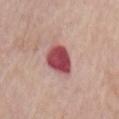follow-up: catalogued during a skin exam; not biopsied
imaging modality: ~15 mm crop, total-body skin-cancer survey
site: the arm
lesion size: about 4 mm
TBP lesion metrics: an area of roughly 10 mm² and a shape-asymmetry score of about 0.2 (0 = symmetric); a classifier nevus-likeness of about 0/100 and a lesion-detection confidence of about 100/100
patient: male, aged 73–77
illumination: white-light illumination